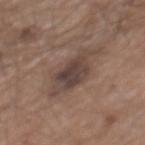Clinical impression: Recorded during total-body skin imaging; not selected for excision or biopsy. Context: About 6 mm across. A male subject roughly 65 years of age. The tile uses white-light illumination. A lesion tile, about 15 mm wide, cut from a 3D total-body photograph. Located on the back.